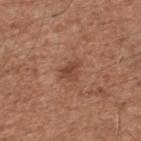workup — imaged on a skin check; not biopsied.